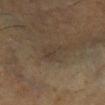Q: Was a biopsy performed?
A: total-body-photography surveillance lesion; no biopsy
Q: What is the anatomic site?
A: the left lower leg
Q: Who is the patient?
A: male, in their 60s
Q: Automated lesion metrics?
A: a lesion color around L≈34 a*≈10 b*≈21 in CIELAB and a lesion–skin lightness drop of about 5
Q: Illumination type?
A: cross-polarized illumination
Q: What kind of image is this?
A: 15 mm crop, total-body photography